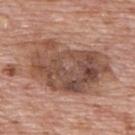Impression:
Part of a total-body skin-imaging series; this lesion was reviewed on a skin check and was not flagged for biopsy.
Image and clinical context:
The subject is a male approximately 70 years of age. Automated tile analysis of the lesion measured a classifier nevus-likeness of about 0/100 and a detector confidence of about 100 out of 100 that the crop contains a lesion. The lesion is located on the upper back. This image is a 15 mm lesion crop taken from a total-body photograph. Captured under white-light illumination. Measured at roughly 9.5 mm in maximum diameter.